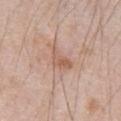Q: Is there a histopathology result?
A: imaged on a skin check; not biopsied
Q: Automated lesion metrics?
A: an average lesion color of about L≈59 a*≈20 b*≈29 (CIELAB), about 8 CIELAB-L* units darker than the surrounding skin, and a lesion-to-skin contrast of about 6 (normalized; higher = more distinct); border irregularity of about 5 on a 0–10 scale, internal color variation of about 2.5 on a 0–10 scale, and radial color variation of about 1; an automated nevus-likeness rating near 0 out of 100 and lesion-presence confidence of about 100/100
Q: What is the anatomic site?
A: the chest
Q: What kind of image is this?
A: ~15 mm tile from a whole-body skin photo
Q: What is the lesion's diameter?
A: ~3.5 mm (longest diameter)
Q: What are the patient's age and sex?
A: male, aged 68–72